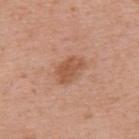Clinical impression:
This lesion was catalogued during total-body skin photography and was not selected for biopsy.
Image and clinical context:
The lesion-visualizer software estimated a footprint of about 7 mm², an eccentricity of roughly 0.8, and two-axis asymmetry of about 0.25. It also reported border irregularity of about 2.5 on a 0–10 scale, internal color variation of about 2 on a 0–10 scale, and radial color variation of about 0.5. Captured under white-light illumination. A region of skin cropped from a whole-body photographic capture, roughly 15 mm wide. The lesion is located on the back. A male patient, aged approximately 60. The lesion's longest dimension is about 3.5 mm.